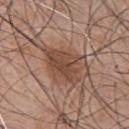Imaged during a routine full-body skin examination; the lesion was not biopsied and no histopathology is available. A lesion tile, about 15 mm wide, cut from a 3D total-body photograph. This is a white-light tile. From the chest. A male patient, about 45 years old.